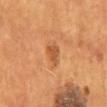Q: Was this lesion biopsied?
A: imaged on a skin check; not biopsied
Q: What is the lesion's diameter?
A: about 2.5 mm
Q: How was this image acquired?
A: ~15 mm tile from a whole-body skin photo
Q: How was the tile lit?
A: cross-polarized
Q: What is the anatomic site?
A: the mid back
Q: Patient demographics?
A: male, approximately 55 years of age